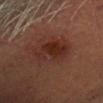Clinical impression: Imaged during a routine full-body skin examination; the lesion was not biopsied and no histopathology is available. Context: The recorded lesion diameter is about 5.5 mm. An algorithmic analysis of the crop reported border irregularity of about 2.5 on a 0–10 scale and radial color variation of about 1.5. The software also gave a nevus-likeness score of about 35/100 and a lesion-detection confidence of about 100/100. Cropped from a total-body skin-imaging series; the visible field is about 15 mm. The lesion is on the head or neck. A male subject, about 60 years old.